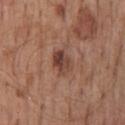| key | value |
|---|---|
| workup | no biopsy performed (imaged during a skin exam) |
| patient | male, about 60 years old |
| TBP lesion metrics | an eccentricity of roughly 0.75; a lesion color around L≈42 a*≈21 b*≈26 in CIELAB, a lesion–skin lightness drop of about 11, and a lesion-to-skin contrast of about 8.5 (normalized; higher = more distinct); a nevus-likeness score of about 55/100 |
| size | ≈3 mm |
| body site | the mid back |
| acquisition | total-body-photography crop, ~15 mm field of view |